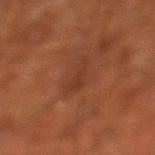Q: What kind of image is this?
A: ~15 mm tile from a whole-body skin photo
Q: Patient demographics?
A: male, roughly 65 years of age
Q: Automated lesion metrics?
A: an average lesion color of about L≈36 a*≈24 b*≈30 (CIELAB), roughly 6 lightness units darker than nearby skin, and a normalized border contrast of about 5.5; border irregularity of about 6 on a 0–10 scale, a within-lesion color-variation index near 1/10, and radial color variation of about 0.5
Q: What is the anatomic site?
A: the left lower leg